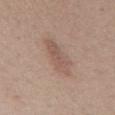notes=catalogued during a skin exam; not biopsied
lesion diameter=about 5.5 mm
body site=the abdomen
tile lighting=white-light
image source=~15 mm tile from a whole-body skin photo
subject=male, in their 40s